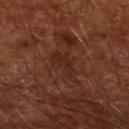Imaged during a routine full-body skin examination; the lesion was not biopsied and no histopathology is available. Located on the left lower leg. The tile uses cross-polarized illumination. A male patient, aged 63 to 67. This image is a 15 mm lesion crop taken from a total-body photograph. Automated image analysis of the tile measured a mean CIELAB color near L≈25 a*≈21 b*≈25 and a normalized border contrast of about 5.5. The analysis additionally found a border-irregularity rating of about 6/10, internal color variation of about 2.5 on a 0–10 scale, and a peripheral color-asymmetry measure near 1. The software also gave a nevus-likeness score of about 0/100. The lesion's longest dimension is about 4.5 mm.